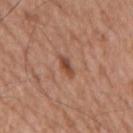follow-up — imaged on a skin check; not biopsied | image — total-body-photography crop, ~15 mm field of view | patient — male, about 75 years old | image-analysis metrics — an area of roughly 2.5 mm² and an outline eccentricity of about 0.9 (0 = round, 1 = elongated); border irregularity of about 2.5 on a 0–10 scale, a color-variation rating of about 2.5/10, and peripheral color asymmetry of about 1 | site — the back | size — ~2.5 mm (longest diameter).